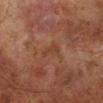Q: Was a biopsy performed?
A: imaged on a skin check; not biopsied
Q: Where on the body is the lesion?
A: the right lower leg
Q: What are the patient's age and sex?
A: male, aged approximately 70
Q: What kind of image is this?
A: ~15 mm tile from a whole-body skin photo